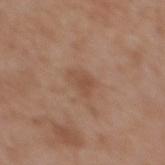The lesion is located on the upper back.
A 15 mm close-up extracted from a 3D total-body photography capture.
The subject is a female aged 33–37.